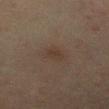Q: How large is the lesion?
A: ~3 mm (longest diameter)
Q: Patient demographics?
A: female, aged 53–57
Q: Illumination type?
A: cross-polarized
Q: How was this image acquired?
A: total-body-photography crop, ~15 mm field of view
Q: Where on the body is the lesion?
A: the right lower leg